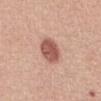biopsy_status: not biopsied; imaged during a skin examination
patient:
  sex: female
  age_approx: 55
automated_metrics:
  cielab_L: 55
  cielab_a: 25
  cielab_b: 27
  vs_skin_darker_L: 15.0
  border_irregularity_0_10: 1.0
  color_variation_0_10: 3.0
  peripheral_color_asymmetry: 1.0
  nevus_likeness_0_100: 100
site: abdomen
lesion_size:
  long_diameter_mm_approx: 3.5
lighting: white-light
image:
  source: total-body photography crop
  field_of_view_mm: 15